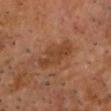<record>
<biopsy_status>not biopsied; imaged during a skin examination</biopsy_status>
<image>
  <source>total-body photography crop</source>
  <field_of_view_mm>15</field_of_view_mm>
</image>
<automated_metrics>
  <cielab_L>39</cielab_L>
  <cielab_a>21</cielab_a>
  <cielab_b>31</cielab_b>
  <vs_skin_darker_L>7.0</vs_skin_darker_L>
  <vs_skin_contrast_norm>6.0</vs_skin_contrast_norm>
</automated_metrics>
<site>head or neck</site>
<lesion_size>
  <long_diameter_mm_approx>4.5</long_diameter_mm_approx>
</lesion_size>
<lighting>cross-polarized</lighting>
<patient>
  <sex>male</sex>
  <age_approx>65</age_approx>
</patient>
</record>